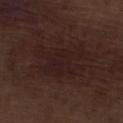No biopsy was performed on this lesion — it was imaged during a full skin examination and was not determined to be concerning. From the left lower leg. This is a white-light tile. Measured at roughly 6 mm in maximum diameter. A region of skin cropped from a whole-body photographic capture, roughly 15 mm wide. A male patient, roughly 70 years of age.